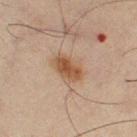| key | value |
|---|---|
| notes | no biopsy performed (imaged during a skin exam) |
| site | the left thigh |
| patient | male, aged approximately 65 |
| illumination | cross-polarized |
| lesion size | about 4 mm |
| automated lesion analysis | a footprint of about 8.5 mm² and a shape-asymmetry score of about 0.2 (0 = symmetric); roughly 9 lightness units darker than nearby skin and a normalized border contrast of about 8.5; a color-variation rating of about 4/10 and a peripheral color-asymmetry measure near 1.5; lesion-presence confidence of about 100/100 |
| image source | ~15 mm tile from a whole-body skin photo |